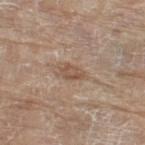Assessment:
Part of a total-body skin-imaging series; this lesion was reviewed on a skin check and was not flagged for biopsy.